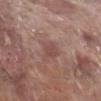Q: Is there a histopathology result?
A: no biopsy performed (imaged during a skin exam)
Q: How was this image acquired?
A: ~15 mm tile from a whole-body skin photo
Q: Who is the patient?
A: male, aged 68 to 72
Q: Lesion location?
A: the right lower leg
Q: How large is the lesion?
A: ≈2.5 mm
Q: Automated lesion metrics?
A: a lesion area of about 3.5 mm², an outline eccentricity of about 0.7 (0 = round, 1 = elongated), and two-axis asymmetry of about 0.3
Q: Illumination type?
A: white-light